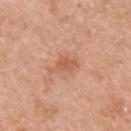<record>
<biopsy_status>not biopsied; imaged during a skin examination</biopsy_status>
<site>left upper arm</site>
<patient>
  <sex>female</sex>
  <age_approx>40</age_approx>
</patient>
<image>
  <source>total-body photography crop</source>
  <field_of_view_mm>15</field_of_view_mm>
</image>
<lighting>white-light</lighting>
<lesion_size>
  <long_diameter_mm_approx>3.5</long_diameter_mm_approx>
</lesion_size>
</record>